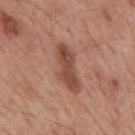tile lighting: white-light illumination; diameter: ~6 mm (longest diameter); location: the mid back; image: 15 mm crop, total-body photography; patient: male, in their mid-50s.